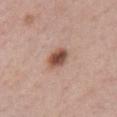Clinical impression:
No biopsy was performed on this lesion — it was imaged during a full skin examination and was not determined to be concerning.
Context:
On the chest. A female subject, aged approximately 40. About 3.5 mm across. This is a white-light tile. This image is a 15 mm lesion crop taken from a total-body photograph.